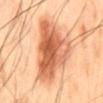Assessment:
Captured during whole-body skin photography for melanoma surveillance; the lesion was not biopsied.
Acquisition and patient details:
From the mid back. A male patient aged around 45. The tile uses cross-polarized illumination. Cropped from a total-body skin-imaging series; the visible field is about 15 mm. Approximately 8.5 mm at its widest.